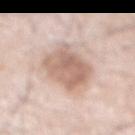– workup · no biopsy performed (imaged during a skin exam)
– image · ~15 mm crop, total-body skin-cancer survey
– body site · the abdomen
– TBP lesion metrics · a lesion area of about 20 mm², an eccentricity of roughly 0.65, and a symmetry-axis asymmetry near 0.25; a mean CIELAB color near L≈65 a*≈17 b*≈26, roughly 12 lightness units darker than nearby skin, and a normalized border contrast of about 7.5; a border-irregularity rating of about 2.5/10 and internal color variation of about 4.5 on a 0–10 scale; an automated nevus-likeness rating near 15 out of 100 and a lesion-detection confidence of about 100/100
– subject · male, aged 78–82
– lighting · white-light illumination